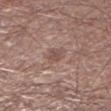Assessment:
This lesion was catalogued during total-body skin photography and was not selected for biopsy.
Acquisition and patient details:
A 15 mm close-up extracted from a 3D total-body photography capture. The subject is a male aged 68–72. On the left lower leg.